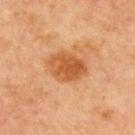Impression:
Part of a total-body skin-imaging series; this lesion was reviewed on a skin check and was not flagged for biopsy.
Image and clinical context:
A roughly 15 mm field-of-view crop from a total-body skin photograph. The lesion is located on the chest. The patient is a male about 65 years old. This is a cross-polarized tile. The lesion's longest dimension is about 4.5 mm.